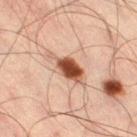{
  "biopsy_status": "not biopsied; imaged during a skin examination",
  "patient": {
    "sex": "male",
    "age_approx": 35
  },
  "site": "right thigh",
  "image": {
    "source": "total-body photography crop",
    "field_of_view_mm": 15
  }
}